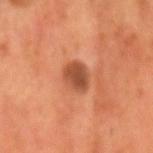{
  "lesion_size": {
    "long_diameter_mm_approx": 3.0
  },
  "site": "head or neck",
  "automated_metrics": {
    "cielab_L": 45,
    "cielab_a": 25,
    "cielab_b": 34,
    "vs_skin_darker_L": 12.0,
    "nevus_likeness_0_100": 60,
    "lesion_detection_confidence_0_100": 100
  },
  "lighting": "cross-polarized",
  "patient": {
    "sex": "male",
    "age_approx": 55
  },
  "image": {
    "source": "total-body photography crop",
    "field_of_view_mm": 15
  }
}